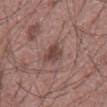biopsy status: catalogued during a skin exam; not biopsied
image-analysis metrics: an area of roughly 6 mm², a shape eccentricity near 0.55, and a symmetry-axis asymmetry near 0.25; a lesion color around L≈44 a*≈19 b*≈21 in CIELAB, about 10 CIELAB-L* units darker than the surrounding skin, and a normalized lesion–skin contrast near 7.5
site: the abdomen
subject: male, roughly 60 years of age
acquisition: ~15 mm tile from a whole-body skin photo
lesion size: ~3 mm (longest diameter)
illumination: white-light